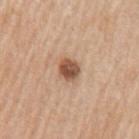Q: Is there a histopathology result?
A: total-body-photography surveillance lesion; no biopsy
Q: What lighting was used for the tile?
A: white-light illumination
Q: Lesion location?
A: the arm
Q: What did automated image analysis measure?
A: a lesion area of about 5 mm², an eccentricity of roughly 0.4, and a shape-asymmetry score of about 0.15 (0 = symmetric); an average lesion color of about L≈52 a*≈21 b*≈31 (CIELAB), roughly 16 lightness units darker than nearby skin, and a lesion-to-skin contrast of about 10.5 (normalized; higher = more distinct); border irregularity of about 1.5 on a 0–10 scale and a color-variation rating of about 4.5/10; a classifier nevus-likeness of about 90/100 and a detector confidence of about 100 out of 100 that the crop contains a lesion
Q: How large is the lesion?
A: ≈2.5 mm
Q: Who is the patient?
A: male, aged around 60
Q: What kind of image is this?
A: ~15 mm crop, total-body skin-cancer survey